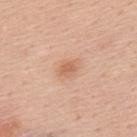Impression:
No biopsy was performed on this lesion — it was imaged during a full skin examination and was not determined to be concerning.
Image and clinical context:
The tile uses white-light illumination. Measured at roughly 3 mm in maximum diameter. A region of skin cropped from a whole-body photographic capture, roughly 15 mm wide. Located on the back. The lesion-visualizer software estimated internal color variation of about 1.5 on a 0–10 scale and radial color variation of about 0.5. The software also gave lesion-presence confidence of about 100/100. A male subject roughly 55 years of age.